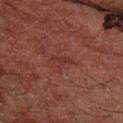The lesion was tiled from a total-body skin photograph and was not biopsied. Imaged with cross-polarized lighting. On the right forearm. A male patient roughly 50 years of age. The lesion's longest dimension is about 3 mm. The lesion-visualizer software estimated a shape eccentricity near 0.9 and a shape-asymmetry score of about 0.35 (0 = symmetric). It also reported a mean CIELAB color near L≈25 a*≈20 b*≈20 and roughly 4 lightness units darker than nearby skin. It also reported a border-irregularity rating of about 4/10, a within-lesion color-variation index near 0.5/10, and peripheral color asymmetry of about 0. The analysis additionally found a lesion-detection confidence of about 100/100. This image is a 15 mm lesion crop taken from a total-body photograph.